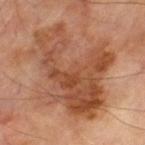Q: Was this lesion biopsied?
A: catalogued during a skin exam; not biopsied
Q: Lesion size?
A: ~10.5 mm (longest diameter)
Q: Who is the patient?
A: male, about 70 years old
Q: What did automated image analysis measure?
A: a lesion area of about 50 mm², a shape eccentricity near 0.65, and a shape-asymmetry score of about 0.4 (0 = symmetric); an average lesion color of about L≈48 a*≈23 b*≈33 (CIELAB); a nevus-likeness score of about 0/100 and lesion-presence confidence of about 100/100
Q: What is the imaging modality?
A: ~15 mm crop, total-body skin-cancer survey
Q: Where on the body is the lesion?
A: the leg
Q: Illumination type?
A: cross-polarized